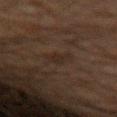The lesion was photographed on a routine skin check and not biopsied; there is no pathology result.
The lesion's longest dimension is about 3 mm.
A male patient, aged approximately 35.
The lesion is on the arm.
A 15 mm close-up tile from a total-body photography series done for melanoma screening.
Captured under cross-polarized illumination.